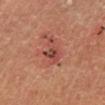Part of a total-body skin-imaging series; this lesion was reviewed on a skin check and was not flagged for biopsy. The patient is a female in their 50s. The tile uses cross-polarized illumination. Automated tile analysis of the lesion measured a lesion color around L≈45 a*≈27 b*≈28 in CIELAB, about 10 CIELAB-L* units darker than the surrounding skin, and a lesion-to-skin contrast of about 7.5 (normalized; higher = more distinct). Measured at roughly 4.5 mm in maximum diameter. This image is a 15 mm lesion crop taken from a total-body photograph.